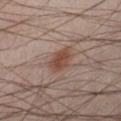{"biopsy_status": "not biopsied; imaged during a skin examination", "image": {"source": "total-body photography crop", "field_of_view_mm": 15}, "site": "left lower leg", "lighting": "cross-polarized", "lesion_size": {"long_diameter_mm_approx": 3.5}, "patient": {"sex": "male", "age_approx": 40}, "automated_metrics": {"cielab_L": 36, "cielab_a": 15, "cielab_b": 21, "vs_skin_darker_L": 8.0, "vs_skin_contrast_norm": 7.5}}